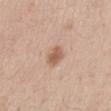{"biopsy_status": "not biopsied; imaged during a skin examination", "lighting": "white-light", "lesion_size": {"long_diameter_mm_approx": 2.5}, "site": "abdomen", "patient": {"sex": "male", "age_approx": 55}, "image": {"source": "total-body photography crop", "field_of_view_mm": 15}}